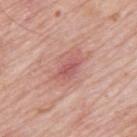Case summary:
– biopsy status: catalogued during a skin exam; not biopsied
– image source: 15 mm crop, total-body photography
– patient: male, aged 78 to 82
– body site: the mid back
– lighting: white-light illumination